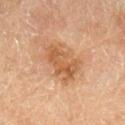* biopsy status: imaged on a skin check; not biopsied
* subject: female, aged 53–57
* TBP lesion metrics: a shape-asymmetry score of about 0.25 (0 = symmetric); a border-irregularity rating of about 3.5/10, a within-lesion color-variation index near 4/10, and radial color variation of about 1.5; an automated nevus-likeness rating near 35 out of 100 and a lesion-detection confidence of about 100/100
* size: ≈5 mm
* acquisition: 15 mm crop, total-body photography
* location: the right lower leg
* tile lighting: cross-polarized illumination